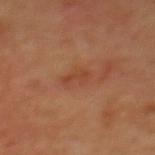Part of a total-body skin-imaging series; this lesion was reviewed on a skin check and was not flagged for biopsy.
A 15 mm close-up tile from a total-body photography series done for melanoma screening.
The lesion is located on the upper back.
A female subject, aged 38–42.